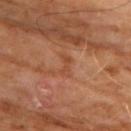Q: Patient demographics?
A: male, in their 60s
Q: What is the imaging modality?
A: total-body-photography crop, ~15 mm field of view
Q: Illumination type?
A: cross-polarized
Q: What is the anatomic site?
A: the front of the torso
Q: How large is the lesion?
A: ≈3 mm